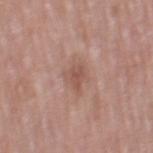Clinical impression:
Part of a total-body skin-imaging series; this lesion was reviewed on a skin check and was not flagged for biopsy.
Image and clinical context:
An algorithmic analysis of the crop reported a footprint of about 4.5 mm² and an eccentricity of roughly 0.6. It also reported about 8 CIELAB-L* units darker than the surrounding skin and a normalized lesion–skin contrast near 6. The patient is a female aged 58–62. From the right thigh. The recorded lesion diameter is about 2.5 mm. A 15 mm crop from a total-body photograph taken for skin-cancer surveillance.